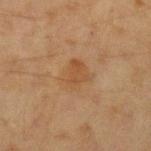Captured during whole-body skin photography for melanoma surveillance; the lesion was not biopsied.
The total-body-photography lesion software estimated an area of roughly 8.5 mm² and a shape-asymmetry score of about 0.3 (0 = symmetric). It also reported a border-irregularity rating of about 3/10, a within-lesion color-variation index near 2.5/10, and radial color variation of about 1. It also reported an automated nevus-likeness rating near 5 out of 100 and a lesion-detection confidence of about 100/100.
Longest diameter approximately 3.5 mm.
A female patient, in their 40s.
The lesion is located on the left forearm.
Captured under cross-polarized illumination.
A region of skin cropped from a whole-body photographic capture, roughly 15 mm wide.